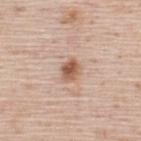A male subject approximately 45 years of age. Automated image analysis of the tile measured a shape eccentricity near 0.65 and a shape-asymmetry score of about 0.2 (0 = symmetric). It also reported a lesion–skin lightness drop of about 13 and a lesion-to-skin contrast of about 9 (normalized; higher = more distinct). And it measured a within-lesion color-variation index near 5/10. It also reported a nevus-likeness score of about 95/100 and a lesion-detection confidence of about 100/100. Located on the upper back. The lesion's longest dimension is about 2.5 mm. A close-up tile cropped from a whole-body skin photograph, about 15 mm across. Imaged with white-light lighting.